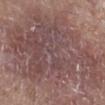Recorded during total-body skin imaging; not selected for excision or biopsy.
The tile uses white-light illumination.
Longest diameter approximately 15.5 mm.
The subject is a male aged 63–67.
A lesion tile, about 15 mm wide, cut from a 3D total-body photograph.
The lesion is on the right lower leg.